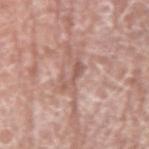Q: Was this lesion biopsied?
A: no biopsy performed (imaged during a skin exam)